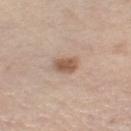| field | value |
|---|---|
| notes | imaged on a skin check; not biopsied |
| anatomic site | the right thigh |
| subject | male, aged 68–72 |
| lesion size | about 3 mm |
| lighting | white-light illumination |
| image source | ~15 mm crop, total-body skin-cancer survey |